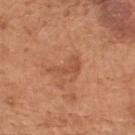Impression: Recorded during total-body skin imaging; not selected for excision or biopsy. Background: Imaged with white-light lighting. The lesion-visualizer software estimated a border-irregularity rating of about 7/10, a color-variation rating of about 1.5/10, and peripheral color asymmetry of about 0.5. Cropped from a whole-body photographic skin survey; the tile spans about 15 mm. The lesion is located on the back. The lesion's longest dimension is about 4 mm. The subject is a male aged around 65.